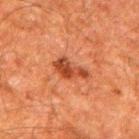Clinical impression:
Recorded during total-body skin imaging; not selected for excision or biopsy.
Image and clinical context:
A male patient, about 60 years old. Automated image analysis of the tile measured a footprint of about 6.5 mm² and two-axis asymmetry of about 0.35. It also reported roughly 10 lightness units darker than nearby skin and a lesion-to-skin contrast of about 8.5 (normalized; higher = more distinct). And it measured a border-irregularity index near 4.5/10, a within-lesion color-variation index near 4/10, and a peripheral color-asymmetry measure near 1.5. Captured under cross-polarized illumination. A region of skin cropped from a whole-body photographic capture, roughly 15 mm wide. Located on the right upper arm.